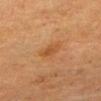  biopsy_status: not biopsied; imaged during a skin examination
  lighting: cross-polarized
  lesion_size:
    long_diameter_mm_approx: 2.5
  automated_metrics:
    area_mm2_approx: 3.5
    eccentricity: 0.8
    shape_asymmetry: 0.2
    cielab_L: 44
    cielab_a: 22
    cielab_b: 37
    vs_skin_darker_L: 6.0
    vs_skin_contrast_norm: 6.0
    nevus_likeness_0_100: 45
  patient:
    sex: male
    age_approx: 85+
  site: head or neck
  image:
    source: total-body photography crop
    field_of_view_mm: 15